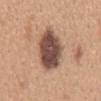Impression:
Captured during whole-body skin photography for melanoma surveillance; the lesion was not biopsied.
Image and clinical context:
An algorithmic analysis of the crop reported a mean CIELAB color near L≈49 a*≈19 b*≈25, roughly 19 lightness units darker than nearby skin, and a normalized lesion–skin contrast near 13. The software also gave a border-irregularity index near 2.5/10, internal color variation of about 4.5 on a 0–10 scale, and a peripheral color-asymmetry measure near 1.5. Measured at roughly 7.5 mm in maximum diameter. From the mid back. A female subject, in their 30s. A lesion tile, about 15 mm wide, cut from a 3D total-body photograph. This is a white-light tile.